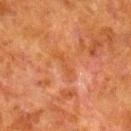| feature | finding |
|---|---|
| workup | catalogued during a skin exam; not biopsied |
| lesion diameter | ≈4 mm |
| tile lighting | cross-polarized illumination |
| image | ~15 mm crop, total-body skin-cancer survey |
| patient | male, aged approximately 80 |
| automated metrics | an outline eccentricity of about 0.9 (0 = round, 1 = elongated) and a shape-asymmetry score of about 0.35 (0 = symmetric); a border-irregularity index near 4.5/10, a color-variation rating of about 1/10, and radial color variation of about 0.5 |
| site | the right lower leg |